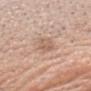Assessment:
The lesion was photographed on a routine skin check and not biopsied; there is no pathology result.
Context:
Imaged with white-light lighting. The total-body-photography lesion software estimated a shape eccentricity near 0.7 and a symmetry-axis asymmetry near 0.25. And it measured a color-variation rating of about 3.5/10. The software also gave a nevus-likeness score of about 0/100 and lesion-presence confidence of about 100/100. On the head or neck. A lesion tile, about 15 mm wide, cut from a 3D total-body photograph. Longest diameter approximately 3 mm. A male patient, aged 58–62.